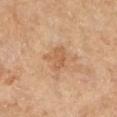{
  "biopsy_status": "not biopsied; imaged during a skin examination",
  "automated_metrics": {
    "border_irregularity_0_10": 4.5,
    "color_variation_0_10": 2.0,
    "peripheral_color_asymmetry": 1.0
  },
  "image": {
    "source": "total-body photography crop",
    "field_of_view_mm": 15
  },
  "lesion_size": {
    "long_diameter_mm_approx": 3.0
  },
  "patient": {
    "sex": "female",
    "age_approx": 65
  },
  "lighting": "cross-polarized",
  "site": "right forearm"
}